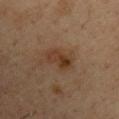biopsy_status: not biopsied; imaged during a skin examination
lesion_size:
  long_diameter_mm_approx: 3.5
automated_metrics:
  cielab_L: 33
  cielab_a: 17
  cielab_b: 27
  vs_skin_contrast_norm: 7.5
  border_irregularity_0_10: 3.0
  color_variation_0_10: 5.0
  peripheral_color_asymmetry: 2.0
site: upper back
image:
  source: total-body photography crop
  field_of_view_mm: 15
patient:
  sex: male
  age_approx: 35